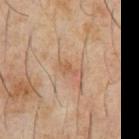  biopsy_status: not biopsied; imaged during a skin examination
  lighting: cross-polarized
  automated_metrics:
    shape_asymmetry: 0.45
    border_irregularity_0_10: 5.5
    color_variation_0_10: 0.5
    peripheral_color_asymmetry: 0.0
    nevus_likeness_0_100: 85
    lesion_detection_confidence_0_100: 100
  site: front of the torso
  image:
    source: total-body photography crop
    field_of_view_mm: 15
  patient:
    sex: male
    age_approx: 60
  lesion_size:
    long_diameter_mm_approx: 3.0